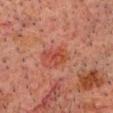<lesion>
<biopsy_status>not biopsied; imaged during a skin examination</biopsy_status>
<automated_metrics>
  <area_mm2_approx>4.0</area_mm2_approx>
  <eccentricity>0.75</eccentricity>
  <shape_asymmetry>0.35</shape_asymmetry>
  <cielab_L>36</cielab_L>
  <cielab_a>26</cielab_a>
  <cielab_b>26</cielab_b>
  <vs_skin_darker_L>6.0</vs_skin_darker_L>
  <vs_skin_contrast_norm>6.5</vs_skin_contrast_norm>
</automated_metrics>
<image>
  <source>total-body photography crop</source>
  <field_of_view_mm>15</field_of_view_mm>
</image>
<site>head or neck</site>
<lesion_size>
  <long_diameter_mm_approx>3.0</long_diameter_mm_approx>
</lesion_size>
<lighting>cross-polarized</lighting>
<patient>
  <sex>male</sex>
  <age_approx>60</age_approx>
</patient>
</lesion>